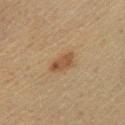biopsy status: catalogued during a skin exam; not biopsied | body site: the left lower leg | subject: male, in their mid-40s | image-analysis metrics: an area of roughly 4.5 mm² and an outline eccentricity of about 0.85 (0 = round, 1 = elongated); a classifier nevus-likeness of about 85/100 and a detector confidence of about 100 out of 100 that the crop contains a lesion | image: ~15 mm tile from a whole-body skin photo | lesion diameter: about 3 mm.